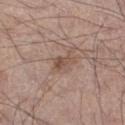The lesion was photographed on a routine skin check and not biopsied; there is no pathology result.
A 15 mm crop from a total-body photograph taken for skin-cancer surveillance.
This is a white-light tile.
The recorded lesion diameter is about 2.5 mm.
Automated tile analysis of the lesion measured a footprint of about 3 mm², an eccentricity of roughly 0.8, and a shape-asymmetry score of about 0.35 (0 = symmetric). The analysis additionally found a mean CIELAB color near L≈50 a*≈18 b*≈26, a lesion–skin lightness drop of about 9, and a lesion-to-skin contrast of about 7 (normalized; higher = more distinct). The analysis additionally found border irregularity of about 3.5 on a 0–10 scale and a peripheral color-asymmetry measure near 1. And it measured an automated nevus-likeness rating near 0 out of 100.
The subject is a male about 55 years old.
From the left thigh.